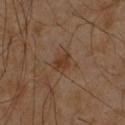Clinical impression: The lesion was photographed on a routine skin check and not biopsied; there is no pathology result. Image and clinical context: A male patient aged 58–62. Longest diameter approximately 2.5 mm. This image is a 15 mm lesion crop taken from a total-body photograph. Located on the chest. Imaged with cross-polarized lighting.